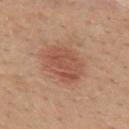The lesion is on the back.
Cropped from a total-body skin-imaging series; the visible field is about 15 mm.
The subject is a female about 45 years old.
Measured at roughly 4.5 mm in maximum diameter.
Imaged with white-light lighting.